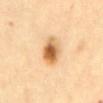Q: Was this lesion biopsied?
A: total-body-photography surveillance lesion; no biopsy
Q: Patient demographics?
A: female, aged approximately 60
Q: What kind of image is this?
A: 15 mm crop, total-body photography
Q: Where on the body is the lesion?
A: the front of the torso